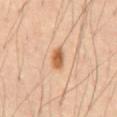Assessment: The lesion was photographed on a routine skin check and not biopsied; there is no pathology result. Background: A lesion tile, about 15 mm wide, cut from a 3D total-body photograph. The patient is a male approximately 60 years of age. The lesion is located on the abdomen.